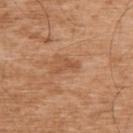The lesion was photographed on a routine skin check and not biopsied; there is no pathology result.
A 15 mm crop from a total-body photograph taken for skin-cancer surveillance.
The patient is a male roughly 75 years of age.
Approximately 2.5 mm at its widest.
This is a white-light tile.
The lesion is located on the back.